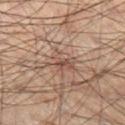Assessment:
Captured during whole-body skin photography for melanoma surveillance; the lesion was not biopsied.
Acquisition and patient details:
A 15 mm close-up extracted from a 3D total-body photography capture. Measured at roughly 4 mm in maximum diameter. A male patient, roughly 65 years of age. On the left thigh. The tile uses cross-polarized illumination.